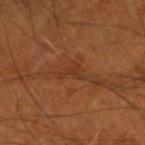Clinical impression:
The lesion was tiled from a total-body skin photograph and was not biopsied.